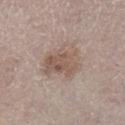Impression:
Part of a total-body skin-imaging series; this lesion was reviewed on a skin check and was not flagged for biopsy.
Clinical summary:
From the right lower leg. A male patient, aged approximately 80. Approximately 5.5 mm at its widest. Cropped from a whole-body photographic skin survey; the tile spans about 15 mm. This is a white-light tile.